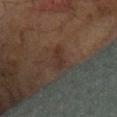Recorded during total-body skin imaging; not selected for excision or biopsy.